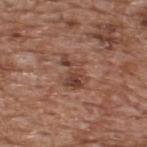notes: catalogued during a skin exam; not biopsied
diameter: about 4 mm
location: the back
imaging modality: 15 mm crop, total-body photography
subject: male, about 65 years old
tile lighting: white-light